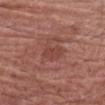The lesion was tiled from a total-body skin photograph and was not biopsied. Cropped from a whole-body photographic skin survey; the tile spans about 15 mm. This is a white-light tile. The total-body-photography lesion software estimated an eccentricity of roughly 0.6 and two-axis asymmetry of about 0.25. It also reported an average lesion color of about L≈43 a*≈25 b*≈25 (CIELAB), roughly 6 lightness units darker than nearby skin, and a lesion-to-skin contrast of about 5 (normalized; higher = more distinct). The lesion is on the arm. Approximately 2.5 mm at its widest. The subject is a male approximately 80 years of age.